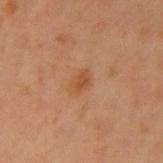  biopsy_status: not biopsied; imaged during a skin examination
  image:
    source: total-body photography crop
    field_of_view_mm: 15
  lesion_size:
    long_diameter_mm_approx: 3.0
  site: left upper arm
  patient:
    sex: male
    age_approx: 60